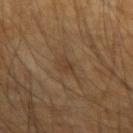lesion size: ≈3.5 mm | automated lesion analysis: an area of roughly 5 mm² and a shape-asymmetry score of about 0.35 (0 = symmetric) | anatomic site: the left forearm | subject: male, about 55 years old | imaging modality: ~15 mm crop, total-body skin-cancer survey.